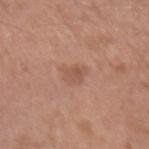Impression:
Imaged during a routine full-body skin examination; the lesion was not biopsied and no histopathology is available.
Background:
The lesion is on the left upper arm. A lesion tile, about 15 mm wide, cut from a 3D total-body photograph. Captured under white-light illumination. The recorded lesion diameter is about 2.5 mm. The subject is a male about 50 years old.